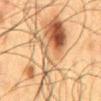Q: Was a biopsy performed?
A: no biopsy performed (imaged during a skin exam)
Q: What is the imaging modality?
A: ~15 mm crop, total-body skin-cancer survey
Q: Automated lesion metrics?
A: a border-irregularity index near 9/10, internal color variation of about 10 on a 0–10 scale, and peripheral color asymmetry of about 3.5
Q: What are the patient's age and sex?
A: male, roughly 60 years of age
Q: How large is the lesion?
A: about 14.5 mm
Q: Lesion location?
A: the mid back